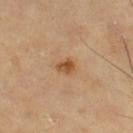No biopsy was performed on this lesion — it was imaged during a full skin examination and was not determined to be concerning.
About 2 mm across.
A 15 mm crop from a total-body photograph taken for skin-cancer surveillance.
A male patient, in their mid-60s.
On the leg.
The tile uses cross-polarized illumination.